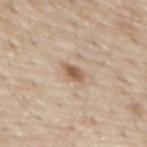Assessment:
The lesion was photographed on a routine skin check and not biopsied; there is no pathology result.
Clinical summary:
This is a white-light tile. From the abdomen. A male patient approximately 70 years of age. Approximately 2.5 mm at its widest. A 15 mm crop from a total-body photograph taken for skin-cancer surveillance.